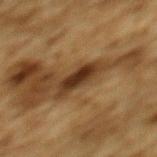Captured during whole-body skin photography for melanoma surveillance; the lesion was not biopsied. From the upper back. A male subject about 85 years old or older. Cropped from a whole-body photographic skin survey; the tile spans about 15 mm. About 4.5 mm across.